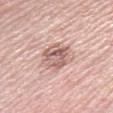notes: total-body-photography surveillance lesion; no biopsy
image source: total-body-photography crop, ~15 mm field of view
subject: female, roughly 65 years of age
anatomic site: the right lower leg